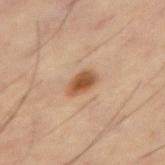Acquisition and patient details:
A 15 mm close-up extracted from a 3D total-body photography capture. On the right thigh. The lesion-visualizer software estimated a footprint of about 5 mm², an eccentricity of roughly 0.8, and a shape-asymmetry score of about 0.15 (0 = symmetric). The software also gave an automated nevus-likeness rating near 95 out of 100 and a detector confidence of about 100 out of 100 that the crop contains a lesion. A male subject aged approximately 60. Longest diameter approximately 3 mm.